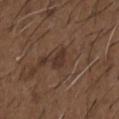<case>
  <biopsy_status>not biopsied; imaged during a skin examination</biopsy_status>
  <site>chest</site>
  <patient>
    <sex>male</sex>
    <age_approx>50</age_approx>
  </patient>
  <automated_metrics>
    <area_mm2_approx>3.5</area_mm2_approx>
    <eccentricity>0.8</eccentricity>
    <cielab_L>32</cielab_L>
    <cielab_a>16</cielab_a>
    <cielab_b>23</cielab_b>
    <vs_skin_darker_L>7.0</vs_skin_darker_L>
    <vs_skin_contrast_norm>7.5</vs_skin_contrast_norm>
    <border_irregularity_0_10>2.5</border_irregularity_0_10>
    <color_variation_0_10>1.5</color_variation_0_10>
  </automated_metrics>
  <lesion_size>
    <long_diameter_mm_approx>2.5</long_diameter_mm_approx>
  </lesion_size>
  <image>
    <source>total-body photography crop</source>
    <field_of_view_mm>15</field_of_view_mm>
  </image>
</case>